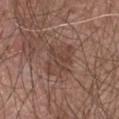Captured during whole-body skin photography for melanoma surveillance; the lesion was not biopsied.
Longest diameter approximately 4 mm.
A male patient, about 65 years old.
A region of skin cropped from a whole-body photographic capture, roughly 15 mm wide.
Located on the chest.
The tile uses white-light illumination.